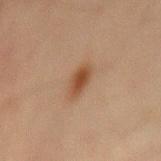<case>
  <biopsy_status>not biopsied; imaged during a skin examination</biopsy_status>
  <site>abdomen</site>
  <image>
    <source>total-body photography crop</source>
    <field_of_view_mm>15</field_of_view_mm>
  </image>
  <patient>
    <sex>male</sex>
    <age_approx>70</age_approx>
  </patient>
</case>